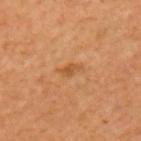This lesion was catalogued during total-body skin photography and was not selected for biopsy.
A 15 mm close-up tile from a total-body photography series done for melanoma screening.
The tile uses cross-polarized illumination.
The lesion-visualizer software estimated a lesion area of about 3 mm², a shape eccentricity near 0.9, and a shape-asymmetry score of about 0.4 (0 = symmetric). It also reported a mean CIELAB color near L≈50 a*≈23 b*≈40, a lesion–skin lightness drop of about 7, and a normalized lesion–skin contrast near 6. The software also gave a border-irregularity rating of about 4.5/10, a color-variation rating of about 1.5/10, and peripheral color asymmetry of about 0.5. The analysis additionally found a classifier nevus-likeness of about 5/100.
On the left upper arm.
About 3 mm across.
A male subject in their 60s.